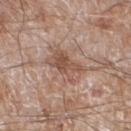The lesion was photographed on a routine skin check and not biopsied; there is no pathology result. The tile uses white-light illumination. The lesion's longest dimension is about 5 mm. On the left lower leg. A roughly 15 mm field-of-view crop from a total-body skin photograph. A male subject aged 58–62.